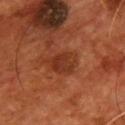No biopsy was performed on this lesion — it was imaged during a full skin examination and was not determined to be concerning.
Located on the chest.
A male patient aged 53 to 57.
A 15 mm close-up tile from a total-body photography series done for melanoma screening.